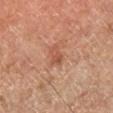Captured during whole-body skin photography for melanoma surveillance; the lesion was not biopsied. The tile uses cross-polarized illumination. A 15 mm close-up tile from a total-body photography series done for melanoma screening. The recorded lesion diameter is about 2.5 mm. Located on the right lower leg. A subject about 65 years old.